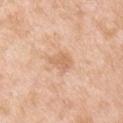Q: Is there a histopathology result?
A: total-body-photography surveillance lesion; no biopsy
Q: What lighting was used for the tile?
A: white-light illumination
Q: Lesion location?
A: the right upper arm
Q: What kind of image is this?
A: ~15 mm tile from a whole-body skin photo
Q: What are the patient's age and sex?
A: male, approximately 55 years of age
Q: Lesion size?
A: ≈3 mm